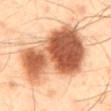  biopsy_status: not biopsied; imaged during a skin examination
  patient:
    sex: male
    age_approx: 40
  site: mid back
  lesion_size:
    long_diameter_mm_approx: 10.5
  lighting: cross-polarized
  image:
    source: total-body photography crop
    field_of_view_mm: 15